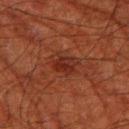The lesion was photographed on a routine skin check and not biopsied; there is no pathology result.
A male subject, roughly 80 years of age.
A 15 mm crop from a total-body photograph taken for skin-cancer surveillance.
The recorded lesion diameter is about 3.5 mm.
On the left thigh.